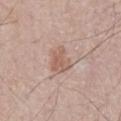<lesion>
<lighting>white-light</lighting>
<patient>
  <sex>male</sex>
  <age_approx>55</age_approx>
</patient>
<image>
  <source>total-body photography crop</source>
  <field_of_view_mm>15</field_of_view_mm>
</image>
<lesion_size>
  <long_diameter_mm_approx>3.0</long_diameter_mm_approx>
</lesion_size>
</lesion>